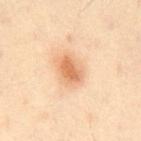Recorded during total-body skin imaging; not selected for excision or biopsy.
The lesion's longest dimension is about 3.5 mm.
A close-up tile cropped from a whole-body skin photograph, about 15 mm across.
Located on the front of the torso.
The subject is a male aged 43–47.
An algorithmic analysis of the crop reported a lesion area of about 7 mm², a shape eccentricity near 0.75, and a symmetry-axis asymmetry near 0.2. The analysis additionally found a border-irregularity index near 1.5/10, internal color variation of about 3.5 on a 0–10 scale, and radial color variation of about 1. It also reported a detector confidence of about 100 out of 100 that the crop contains a lesion.